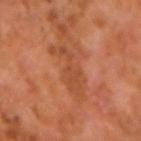Q: Illumination type?
A: cross-polarized
Q: How was this image acquired?
A: ~15 mm tile from a whole-body skin photo
Q: Patient demographics?
A: male, roughly 60 years of age
Q: What is the lesion's diameter?
A: ≈5.5 mm
Q: What is the anatomic site?
A: the left forearm